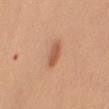Clinical summary:
A male subject aged around 50. About 3 mm across. Cropped from a whole-body photographic skin survey; the tile spans about 15 mm. The total-body-photography lesion software estimated a color-variation rating of about 2.5/10 and peripheral color asymmetry of about 1. Located on the arm.